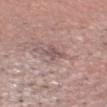– subject: male, roughly 60 years of age
– tile lighting: white-light
– automated metrics: a mean CIELAB color near L≈53 a*≈17 b*≈19 and about 8 CIELAB-L* units darker than the surrounding skin
– site: the head or neck
– image: total-body-photography crop, ~15 mm field of view
– size: ~2.5 mm (longest diameter)
– diagnosis: an invasive melanoma arising in association with a nevus — Breslow thickness 0.5 mm